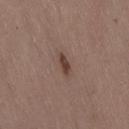{
  "biopsy_status": "not biopsied; imaged during a skin examination",
  "lighting": "white-light",
  "image": {
    "source": "total-body photography crop",
    "field_of_view_mm": 15
  },
  "site": "lower back",
  "lesion_size": {
    "long_diameter_mm_approx": 2.5
  },
  "patient": {
    "sex": "female",
    "age_approx": 50
  }
}